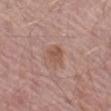Assessment: The lesion was tiled from a total-body skin photograph and was not biopsied. Background: Longest diameter approximately 2.5 mm. Cropped from a whole-body photographic skin survey; the tile spans about 15 mm. Captured under white-light illumination. The patient is a male aged around 65. The lesion is located on the leg.